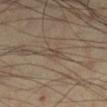Q: Was a biopsy performed?
A: catalogued during a skin exam; not biopsied
Q: Patient demographics?
A: male, in their mid- to late 50s
Q: What is the lesion's diameter?
A: about 3.5 mm
Q: Where on the body is the lesion?
A: the right lower leg
Q: What kind of image is this?
A: ~15 mm tile from a whole-body skin photo